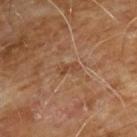Assessment: Part of a total-body skin-imaging series; this lesion was reviewed on a skin check and was not flagged for biopsy. Acquisition and patient details: The lesion's longest dimension is about 2.5 mm. A region of skin cropped from a whole-body photographic capture, roughly 15 mm wide. From the chest. The patient is a male aged 58–62.